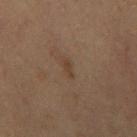workup: imaged on a skin check; not biopsied | subject: male, in their 70s | image-analysis metrics: roughly 4 lightness units darker than nearby skin and a normalized border contrast of about 5.5 | location: the back | acquisition: total-body-photography crop, ~15 mm field of view | tile lighting: cross-polarized | lesion diameter: about 2.5 mm.